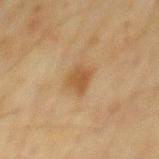biopsy status — catalogued during a skin exam; not biopsied.